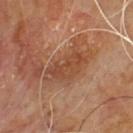Case summary:
– notes: imaged on a skin check; not biopsied